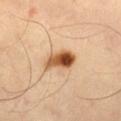Impression:
Part of a total-body skin-imaging series; this lesion was reviewed on a skin check and was not flagged for biopsy.
Background:
A male subject, in their mid-50s. An algorithmic analysis of the crop reported a lesion area of about 7.5 mm², a shape eccentricity near 0.85, and a symmetry-axis asymmetry near 0.25. The analysis additionally found a mean CIELAB color near L≈52 a*≈22 b*≈37 and a lesion–skin lightness drop of about 19. The software also gave peripheral color asymmetry of about 5.5. It also reported a classifier nevus-likeness of about 100/100. A 15 mm close-up extracted from a 3D total-body photography capture. Located on the left thigh. This is a cross-polarized tile.